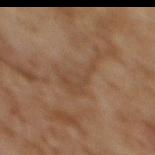biopsy status=imaged on a skin check; not biopsied | illumination=cross-polarized | site=the back | image source=~15 mm tile from a whole-body skin photo | size=≈5.5 mm | patient=female, approximately 60 years of age.